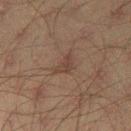Image and clinical context: The subject is a male aged approximately 45. Cropped from a total-body skin-imaging series; the visible field is about 15 mm. The lesion is on the left thigh. Imaged with cross-polarized lighting. Approximately 2.5 mm at its widest.